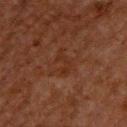Notes:
• notes — total-body-photography surveillance lesion; no biopsy
• patient — male, about 60 years old
• anatomic site — the back
• image — ~15 mm crop, total-body skin-cancer survey